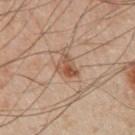| field | value |
|---|---|
| follow-up | imaged on a skin check; not biopsied |
| acquisition | ~15 mm tile from a whole-body skin photo |
| lighting | cross-polarized illumination |
| lesion diameter | ~3.5 mm (longest diameter) |
| location | the left arm |
| subject | male, aged around 50 |